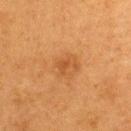notes=catalogued during a skin exam; not biopsied | diameter=about 2.5 mm | image=~15 mm crop, total-body skin-cancer survey | lighting=cross-polarized | site=the upper back | subject=female, roughly 40 years of age | automated metrics=a lesion area of about 3 mm² and a symmetry-axis asymmetry near 0.5; an average lesion color of about L≈42 a*≈23 b*≈37 (CIELAB) and roughly 7 lightness units darker than nearby skin; a border-irregularity index near 5.5/10 and a within-lesion color-variation index near 0.5/10; a nevus-likeness score of about 0/100 and lesion-presence confidence of about 100/100.